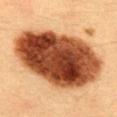No biopsy was performed on this lesion — it was imaged during a full skin examination and was not determined to be concerning.
Imaged with cross-polarized lighting.
Longest diameter approximately 12.5 mm.
Located on the upper back.
The lesion-visualizer software estimated a shape eccentricity near 0.85 and two-axis asymmetry of about 0.1. It also reported a border-irregularity rating of about 1.5/10, a within-lesion color-variation index near 10/10, and peripheral color asymmetry of about 3.5. The software also gave a nevus-likeness score of about 95/100 and a lesion-detection confidence of about 100/100.
The subject is a female in their 30s.
A 15 mm close-up tile from a total-body photography series done for melanoma screening.